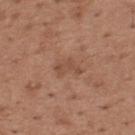Case summary:
– follow-up — total-body-photography surveillance lesion; no biopsy
– subject — male, aged 63–67
– lesion size — ≈3.5 mm
– anatomic site — the upper back
– automated lesion analysis — a border-irregularity rating of about 7/10, a within-lesion color-variation index near 0/10, and a peripheral color-asymmetry measure near 0
– imaging modality — 15 mm crop, total-body photography